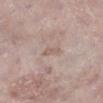  biopsy_status: not biopsied; imaged during a skin examination
  patient:
    sex: female
    age_approx: 70
  automated_metrics:
    area_mm2_approx: 2.5
    eccentricity: 0.9
    shape_asymmetry: 0.25
    cielab_L: 59
    cielab_a: 15
    cielab_b: 24
    vs_skin_darker_L: 6.0
    vs_skin_contrast_norm: 4.5
    border_irregularity_0_10: 2.5
    color_variation_0_10: 0.0
    peripheral_color_asymmetry: 0.0
    nevus_likeness_0_100: 0
    lesion_detection_confidence_0_100: 75
  lesion_size:
    long_diameter_mm_approx: 2.5
  image:
    source: total-body photography crop
    field_of_view_mm: 15
  site: leg